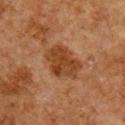| field | value |
|---|---|
| image source | total-body-photography crop, ~15 mm field of view |
| location | the chest |
| patient | male, approximately 60 years of age |